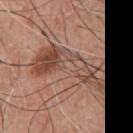This image is a 15 mm lesion crop taken from a total-body photograph.
The total-body-photography lesion software estimated a symmetry-axis asymmetry near 0.6. The analysis additionally found an average lesion color of about L≈48 a*≈20 b*≈27 (CIELAB), a lesion–skin lightness drop of about 10, and a normalized border contrast of about 7.5.
A male patient, roughly 55 years of age.
From the chest.
This is a white-light tile.